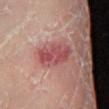biopsy_status: not biopsied; imaged during a skin examination
patient:
  sex: female
  age_approx: 60
image:
  source: total-body photography crop
  field_of_view_mm: 15
site: leg
lesion_size:
  long_diameter_mm_approx: 5.0
lighting: cross-polarized